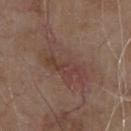workup: imaged on a skin check; not biopsied
patient: male, in their 80s
location: the front of the torso
image: ~15 mm tile from a whole-body skin photo
lesion size: ~8.5 mm (longest diameter)
tile lighting: white-light